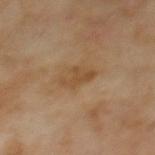notes: total-body-photography surveillance lesion; no biopsy | anatomic site: the mid back | image source: ~15 mm crop, total-body skin-cancer survey | subject: male, approximately 70 years of age.